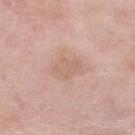A female subject in their 70s. Captured under white-light illumination. This image is a 15 mm lesion crop taken from a total-body photograph. The recorded lesion diameter is about 3.5 mm. From the right lower leg. Automated tile analysis of the lesion measured a footprint of about 7 mm², a shape eccentricity near 0.75, and a symmetry-axis asymmetry near 0.25.